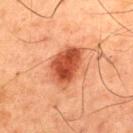biopsy status — total-body-photography surveillance lesion; no biopsy
patient — male, in their 60s
lesion size — about 5 mm
imaging modality — ~15 mm tile from a whole-body skin photo
TBP lesion metrics — a footprint of about 13 mm², an outline eccentricity of about 0.75 (0 = round, 1 = elongated), and a shape-asymmetry score of about 0.2 (0 = symmetric); internal color variation of about 5 on a 0–10 scale and radial color variation of about 2
location — the upper back